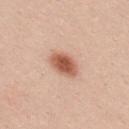Clinical impression:
The lesion was photographed on a routine skin check and not biopsied; there is no pathology result.
Clinical summary:
An algorithmic analysis of the crop reported an area of roughly 7 mm² and a shape-asymmetry score of about 0.2 (0 = symmetric). And it measured a mean CIELAB color near L≈57 a*≈25 b*≈32, a lesion–skin lightness drop of about 16, and a normalized lesion–skin contrast near 10. The software also gave a border-irregularity index near 2/10, a color-variation rating of about 3.5/10, and peripheral color asymmetry of about 1. The analysis additionally found a nevus-likeness score of about 100/100 and lesion-presence confidence of about 100/100. Approximately 3.5 mm at its widest. Cropped from a total-body skin-imaging series; the visible field is about 15 mm. This is a white-light tile. Located on the back. The patient is a female aged 18 to 22.